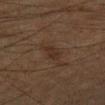Findings:
• workup — imaged on a skin check; not biopsied
• image-analysis metrics — an area of roughly 3 mm², an eccentricity of roughly 0.85, and a symmetry-axis asymmetry near 0.2; a lesion color around L≈22 a*≈13 b*≈20 in CIELAB, roughly 5 lightness units darker than nearby skin, and a normalized lesion–skin contrast near 6.5; a border-irregularity index near 2.5/10 and a within-lesion color-variation index near 0.5/10; an automated nevus-likeness rating near 0 out of 100 and lesion-presence confidence of about 100/100
• tile lighting — cross-polarized illumination
• imaging modality — ~15 mm crop, total-body skin-cancer survey
• subject — male, about 65 years old
• diameter — ≈2.5 mm
• site — the left forearm